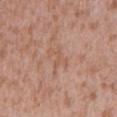Findings:
- workup: total-body-photography surveillance lesion; no biopsy
- patient: male, approximately 45 years of age
- image: total-body-photography crop, ~15 mm field of view
- size: ≈3.5 mm
- anatomic site: the mid back